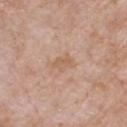Captured during whole-body skin photography for melanoma surveillance; the lesion was not biopsied. Captured under white-light illumination. A male subject aged 58–62. From the chest. A region of skin cropped from a whole-body photographic capture, roughly 15 mm wide.